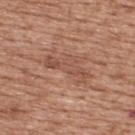| field | value |
|---|---|
| workup | no biopsy performed (imaged during a skin exam) |
| lighting | white-light illumination |
| automated lesion analysis | a mean CIELAB color near L≈51 a*≈22 b*≈29 and a normalized border contrast of about 5.5; a within-lesion color-variation index near 2.5/10; a nevus-likeness score of about 0/100 and a lesion-detection confidence of about 70/100 |
| acquisition | ~15 mm tile from a whole-body skin photo |
| subject | male, aged 58 to 62 |
| lesion size | ~6 mm (longest diameter) |
| location | the upper back |